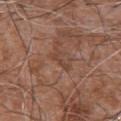Q: Is there a histopathology result?
A: catalogued during a skin exam; not biopsied
Q: Where on the body is the lesion?
A: the front of the torso
Q: Patient demographics?
A: male, approximately 75 years of age
Q: How was this image acquired?
A: ~15 mm tile from a whole-body skin photo
Q: How large is the lesion?
A: about 3 mm